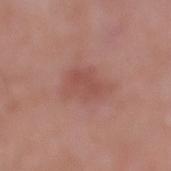  lighting: white-light
  site: right lower leg
  lesion_size:
    long_diameter_mm_approx: 5.0
  patient:
    sex: male
    age_approx: 85+
  automated_metrics:
    cielab_L: 51
    cielab_a: 24
    cielab_b: 25
    vs_skin_darker_L: 7.0
    vs_skin_contrast_norm: 5.0
    border_irregularity_0_10: 3.5
    color_variation_0_10: 2.0
    peripheral_color_asymmetry: 0.5
    nevus_likeness_0_100: 15
    lesion_detection_confidence_0_100: 100
  image:
    source: total-body photography crop
    field_of_view_mm: 15